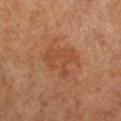Impression: Recorded during total-body skin imaging; not selected for excision or biopsy. Background: A female subject aged around 55. Longest diameter approximately 5 mm. This image is a 15 mm lesion crop taken from a total-body photograph. The total-body-photography lesion software estimated an average lesion color of about L≈50 a*≈26 b*≈36 (CIELAB), roughly 6 lightness units darker than nearby skin, and a lesion-to-skin contrast of about 5 (normalized; higher = more distinct). The software also gave border irregularity of about 5 on a 0–10 scale, internal color variation of about 3 on a 0–10 scale, and radial color variation of about 1. It also reported a classifier nevus-likeness of about 20/100 and a lesion-detection confidence of about 100/100. The tile uses cross-polarized illumination. On the right lower leg.